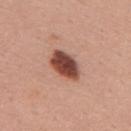Clinical impression: Imaged during a routine full-body skin examination; the lesion was not biopsied and no histopathology is available. Context: The recorded lesion diameter is about 4.5 mm. A 15 mm close-up extracted from a 3D total-body photography capture. On the upper back. A female patient, approximately 55 years of age. Imaged with white-light lighting.